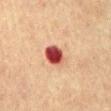Captured during whole-body skin photography for melanoma surveillance; the lesion was not biopsied. The lesion is on the abdomen. This is a cross-polarized tile. A 15 mm close-up extracted from a 3D total-body photography capture. The patient is a male aged 73 to 77.